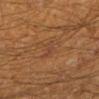| field | value |
|---|---|
| notes | no biopsy performed (imaged during a skin exam) |
| body site | the leg |
| size | about 2.5 mm |
| imaging modality | total-body-photography crop, ~15 mm field of view |
| tile lighting | cross-polarized illumination |
| subject | male, about 60 years old |